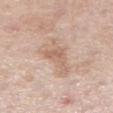Q: Is there a histopathology result?
A: catalogued during a skin exam; not biopsied
Q: Where on the body is the lesion?
A: the leg
Q: Lesion size?
A: about 6 mm
Q: What kind of image is this?
A: 15 mm crop, total-body photography
Q: Automated lesion metrics?
A: a lesion area of about 12 mm²; an average lesion color of about L≈64 a*≈18 b*≈28 (CIELAB)
Q: Patient demographics?
A: female, roughly 65 years of age
Q: How was the tile lit?
A: white-light